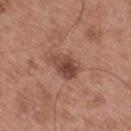Part of a total-body skin-imaging series; this lesion was reviewed on a skin check and was not flagged for biopsy. Captured under white-light illumination. A male patient, in their mid- to late 50s. On the mid back. Approximately 4 mm at its widest. A region of skin cropped from a whole-body photographic capture, roughly 15 mm wide.